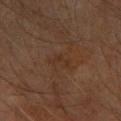Context: Automated image analysis of the tile measured an eccentricity of roughly 0.9 and a shape-asymmetry score of about 0.65 (0 = symmetric). A female subject, approximately 70 years of age. This is a cross-polarized tile. The lesion's longest dimension is about 3 mm. Cropped from a total-body skin-imaging series; the visible field is about 15 mm. The lesion is located on the arm.